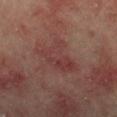• workup: no biopsy performed (imaged during a skin exam)
• lighting: cross-polarized
• subject: male, aged 58–62
• lesion diameter: ≈5 mm
• acquisition: total-body-photography crop, ~15 mm field of view
• anatomic site: the left forearm
• automated metrics: a lesion area of about 14 mm² and an eccentricity of roughly 0.65; about 5 CIELAB-L* units darker than the surrounding skin and a lesion-to-skin contrast of about 5 (normalized; higher = more distinct); an automated nevus-likeness rating near 0 out of 100 and a detector confidence of about 100 out of 100 that the crop contains a lesion